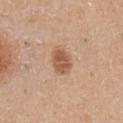workup: imaged on a skin check; not biopsied
patient: male, approximately 40 years of age
image source: 15 mm crop, total-body photography
body site: the chest
lesion diameter: ~3.5 mm (longest diameter)
tile lighting: white-light